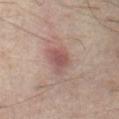Assessment:
Imaged during a routine full-body skin examination; the lesion was not biopsied and no histopathology is available.
Image and clinical context:
Captured under cross-polarized illumination. A male patient, aged approximately 60. A lesion tile, about 15 mm wide, cut from a 3D total-body photograph. The lesion's longest dimension is about 3 mm. The lesion is located on the abdomen.